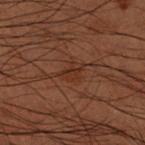Q: What is the imaging modality?
A: 15 mm crop, total-body photography
Q: Illumination type?
A: cross-polarized illumination
Q: Patient demographics?
A: male, roughly 50 years of age
Q: Lesion size?
A: about 2.5 mm
Q: What did automated image analysis measure?
A: a mean CIELAB color near L≈22 a*≈17 b*≈23 and about 5 CIELAB-L* units darker than the surrounding skin
Q: Where on the body is the lesion?
A: the right forearm